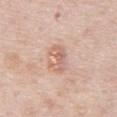workup=catalogued during a skin exam; not biopsied | lesion diameter=~4 mm (longest diameter) | patient=male, aged approximately 75 | site=the abdomen | acquisition=~15 mm tile from a whole-body skin photo | TBP lesion metrics=a lesion area of about 6.5 mm², an eccentricity of roughly 0.85, and a symmetry-axis asymmetry near 0.3; an average lesion color of about L≈64 a*≈21 b*≈28 (CIELAB), about 9 CIELAB-L* units darker than the surrounding skin, and a normalized lesion–skin contrast near 5.5; a border-irregularity rating of about 4.5/10; a detector confidence of about 100 out of 100 that the crop contains a lesion.